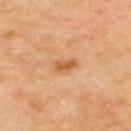Notes:
• follow-up · total-body-photography surveillance lesion; no biopsy
• automated lesion analysis · internal color variation of about 2 on a 0–10 scale and peripheral color asymmetry of about 0.5
• imaging modality · 15 mm crop, total-body photography
• diameter · about 2.5 mm
• lighting · cross-polarized illumination
• location · the upper back